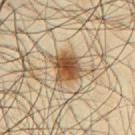| feature | finding |
|---|---|
| follow-up | imaged on a skin check; not biopsied |
| tile lighting | cross-polarized illumination |
| site | the chest |
| lesion diameter | ≈4.5 mm |
| patient | male, aged 63–67 |
| image | total-body-photography crop, ~15 mm field of view |